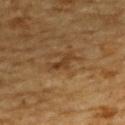This lesion was catalogued during total-body skin photography and was not selected for biopsy.
The lesion is located on the upper back.
The lesion-visualizer software estimated a lesion color around L≈32 a*≈17 b*≈30 in CIELAB and a lesion–skin lightness drop of about 7. The analysis additionally found a border-irregularity rating of about 4.5/10, a within-lesion color-variation index near 0/10, and peripheral color asymmetry of about 0. It also reported a lesion-detection confidence of about 90/100.
The tile uses cross-polarized illumination.
A male subject roughly 85 years of age or older.
The recorded lesion diameter is about 2.5 mm.
Cropped from a whole-body photographic skin survey; the tile spans about 15 mm.